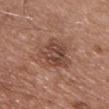- notes: no biopsy performed (imaged during a skin exam)
- subject: male, aged 53–57
- tile lighting: white-light illumination
- body site: the chest
- lesion size: ≈5.5 mm
- image source: total-body-photography crop, ~15 mm field of view